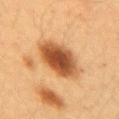Case summary:
– workup — imaged on a skin check; not biopsied
– site — the right upper arm
– image — total-body-photography crop, ~15 mm field of view
– patient — female, aged approximately 40